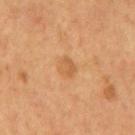This lesion was catalogued during total-body skin photography and was not selected for biopsy. Located on the mid back. The subject is a male aged 53–57. A lesion tile, about 15 mm wide, cut from a 3D total-body photograph. The recorded lesion diameter is about 2.5 mm. The lesion-visualizer software estimated an area of roughly 4 mm².